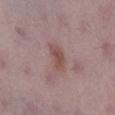{"biopsy_status": "not biopsied; imaged during a skin examination", "image": {"source": "total-body photography crop", "field_of_view_mm": 15}, "automated_metrics": {"color_variation_0_10": 2.5, "peripheral_color_asymmetry": 1.0}, "patient": {"sex": "female", "age_approx": 50}, "lesion_size": {"long_diameter_mm_approx": 3.5}, "site": "right lower leg"}